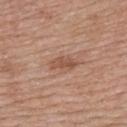Q: Was this lesion biopsied?
A: imaged on a skin check; not biopsied
Q: Where on the body is the lesion?
A: the upper back
Q: How was this image acquired?
A: ~15 mm crop, total-body skin-cancer survey
Q: What are the patient's age and sex?
A: female, aged 48–52
Q: Illumination type?
A: white-light illumination